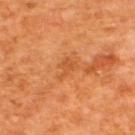Clinical summary:
Measured at roughly 3 mm in maximum diameter. This is a cross-polarized tile. Automated image analysis of the tile measured an eccentricity of roughly 0.8 and a symmetry-axis asymmetry near 0.55. It also reported a border-irregularity index near 6/10, a within-lesion color-variation index near 0.5/10, and radial color variation of about 0.5. The software also gave a detector confidence of about 100 out of 100 that the crop contains a lesion. A male patient, in their mid-60s. From the back. A close-up tile cropped from a whole-body skin photograph, about 15 mm across.